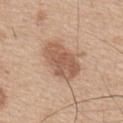Impression:
No biopsy was performed on this lesion — it was imaged during a full skin examination and was not determined to be concerning.
Context:
From the upper back. A roughly 15 mm field-of-view crop from a total-body skin photograph. An algorithmic analysis of the crop reported a lesion area of about 15 mm² and a symmetry-axis asymmetry near 0.15. And it measured an average lesion color of about L≈57 a*≈20 b*≈30 (CIELAB) and a lesion–skin lightness drop of about 11. The subject is a male about 65 years old. Imaged with white-light lighting. Longest diameter approximately 6 mm.